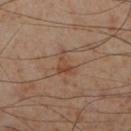Q: Was this lesion biopsied?
A: catalogued during a skin exam; not biopsied
Q: Lesion location?
A: the right lower leg
Q: What lighting was used for the tile?
A: cross-polarized
Q: Patient demographics?
A: male, aged around 55
Q: What kind of image is this?
A: ~15 mm tile from a whole-body skin photo